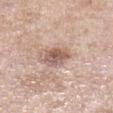| field | value |
|---|---|
| follow-up | no biopsy performed (imaged during a skin exam) |
| imaging modality | total-body-photography crop, ~15 mm field of view |
| lighting | white-light |
| location | the left lower leg |
| patient | female, aged 53–57 |